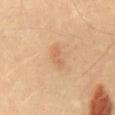Context: Imaged with cross-polarized lighting. Measured at roughly 2.5 mm in maximum diameter. A close-up tile cropped from a whole-body skin photograph, about 15 mm across. The lesion is on the back. The patient is a male aged approximately 40.